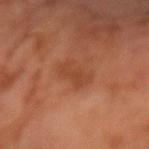Impression:
The lesion was tiled from a total-body skin photograph and was not biopsied.
Clinical summary:
Captured under cross-polarized illumination. A lesion tile, about 15 mm wide, cut from a 3D total-body photograph. A male subject in their mid-60s. Measured at roughly 3.5 mm in maximum diameter.